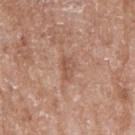Case summary:
– biopsy status · total-body-photography surveillance lesion; no biopsy
– lesion size · ≈3 mm
– site · the right upper arm
– subject · female, aged around 75
– lighting · white-light
– image · ~15 mm tile from a whole-body skin photo